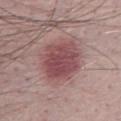Notes:
- biopsy status · catalogued during a skin exam; not biopsied
- TBP lesion metrics · an automated nevus-likeness rating near 80 out of 100 and a lesion-detection confidence of about 100/100
- subject · male, aged approximately 30
- acquisition · total-body-photography crop, ~15 mm field of view